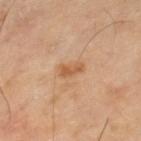<record>
  <biopsy_status>not biopsied; imaged during a skin examination</biopsy_status>
</record>